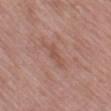The lesion was photographed on a routine skin check and not biopsied; there is no pathology result.
Approximately 3.5 mm at its widest.
A close-up tile cropped from a whole-body skin photograph, about 15 mm across.
Imaged with white-light lighting.
A female patient aged 58 to 62.
The lesion is on the mid back.